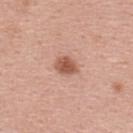Q: Is there a histopathology result?
A: catalogued during a skin exam; not biopsied
Q: Who is the patient?
A: female, aged approximately 40
Q: What is the imaging modality?
A: ~15 mm tile from a whole-body skin photo
Q: What is the anatomic site?
A: the upper back